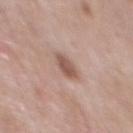Assessment: Captured during whole-body skin photography for melanoma surveillance; the lesion was not biopsied. Context: Located on the front of the torso. The lesion's longest dimension is about 2.5 mm. The total-body-photography lesion software estimated an eccentricity of roughly 0.75 and a shape-asymmetry score of about 0.2 (0 = symmetric). And it measured a lesion color around L≈54 a*≈19 b*≈25 in CIELAB, roughly 11 lightness units darker than nearby skin, and a normalized lesion–skin contrast near 8. The software also gave border irregularity of about 2 on a 0–10 scale and a peripheral color-asymmetry measure near 0.5. The analysis additionally found a nevus-likeness score of about 70/100 and a lesion-detection confidence of about 100/100. A male patient in their mid-50s. This is a white-light tile. Cropped from a whole-body photographic skin survey; the tile spans about 15 mm.